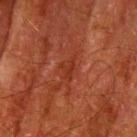This lesion was catalogued during total-body skin photography and was not selected for biopsy. The patient is a male in their 80s. A 15 mm close-up tile from a total-body photography series done for melanoma screening. From the left upper arm. Longest diameter approximately 3 mm. This is a cross-polarized tile. The total-body-photography lesion software estimated an outline eccentricity of about 0.75 (0 = round, 1 = elongated) and a shape-asymmetry score of about 0.5 (0 = symmetric). The software also gave a mean CIELAB color near L≈27 a*≈26 b*≈28 and roughly 5 lightness units darker than nearby skin. It also reported a classifier nevus-likeness of about 0/100 and a detector confidence of about 70 out of 100 that the crop contains a lesion.